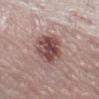Findings:
– follow-up — no biopsy performed (imaged during a skin exam)
– size — ≈5.5 mm
– imaging modality — ~15 mm crop, total-body skin-cancer survey
– patient — female, about 65 years old
– body site — the left thigh
– automated metrics — an area of roughly 15 mm² and an eccentricity of roughly 0.8; about 14 CIELAB-L* units darker than the surrounding skin and a normalized border contrast of about 10